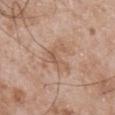follow-up — no biopsy performed (imaged during a skin exam) | imaging modality — ~15 mm tile from a whole-body skin photo | body site — the mid back | lesion size — about 5.5 mm | illumination — white-light illumination | subject — male, aged around 50 | automated metrics — a lesion area of about 11 mm² and an eccentricity of roughly 0.6.